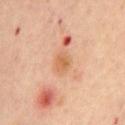Recorded during total-body skin imaging; not selected for excision or biopsy. This is a cross-polarized tile. On the chest. Longest diameter approximately 2.5 mm. A close-up tile cropped from a whole-body skin photograph, about 15 mm across. A female patient roughly 45 years of age.